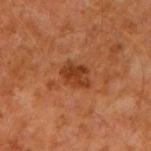The lesion was tiled from a total-body skin photograph and was not biopsied.
A region of skin cropped from a whole-body photographic capture, roughly 15 mm wide.
Automated image analysis of the tile measured a footprint of about 6 mm² and two-axis asymmetry of about 0.3. The analysis additionally found an average lesion color of about L≈37 a*≈26 b*≈35 (CIELAB) and a normalized lesion–skin contrast near 8. And it measured an automated nevus-likeness rating near 70 out of 100.
Located on the right upper arm.
The patient is a male aged 58 to 62.
This is a cross-polarized tile.